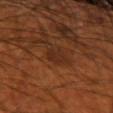The lesion was photographed on a routine skin check and not biopsied; there is no pathology result. The recorded lesion diameter is about 2.5 mm. Automated image analysis of the tile measured a footprint of about 4.5 mm² and a shape eccentricity near 0.5. And it measured a mean CIELAB color near L≈27 a*≈21 b*≈29, about 5 CIELAB-L* units darker than the surrounding skin, and a normalized border contrast of about 6. And it measured a border-irregularity rating of about 2.5/10 and a peripheral color-asymmetry measure near 1. The software also gave a detector confidence of about 100 out of 100 that the crop contains a lesion. Cropped from a total-body skin-imaging series; the visible field is about 15 mm. Captured under cross-polarized illumination. From the arm. A male subject aged 68 to 72.